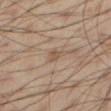workup: imaged on a skin check; not biopsied
site: the right thigh
image source: total-body-photography crop, ~15 mm field of view
patient: male, roughly 55 years of age
TBP lesion metrics: an average lesion color of about L≈53 a*≈15 b*≈28 (CIELAB), about 7 CIELAB-L* units darker than the surrounding skin, and a normalized lesion–skin contrast near 5.5; a border-irregularity index near 2.5/10 and a within-lesion color-variation index near 1.5/10
lesion size: ≈2.5 mm
illumination: cross-polarized illumination